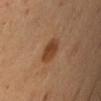follow-up: no biopsy performed (imaged during a skin exam)
TBP lesion metrics: an average lesion color of about L≈42 a*≈21 b*≈34 (CIELAB) and roughly 10 lightness units darker than nearby skin; a classifier nevus-likeness of about 90/100
body site: the left upper arm
patient: male, aged 48 to 52
size: ≈3.5 mm
image: ~15 mm crop, total-body skin-cancer survey
illumination: cross-polarized illumination